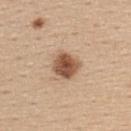Q: Was a biopsy performed?
A: no biopsy performed (imaged during a skin exam)
Q: What lighting was used for the tile?
A: white-light
Q: Patient demographics?
A: female, in their mid-40s
Q: What kind of image is this?
A: 15 mm crop, total-body photography
Q: What is the lesion's diameter?
A: ≈3.5 mm
Q: What did automated image analysis measure?
A: a mean CIELAB color near L≈54 a*≈20 b*≈32 and a lesion–skin lightness drop of about 16; a border-irregularity rating of about 1.5/10, a color-variation rating of about 4.5/10, and peripheral color asymmetry of about 1.5; an automated nevus-likeness rating near 100 out of 100 and a detector confidence of about 100 out of 100 that the crop contains a lesion
Q: What is the anatomic site?
A: the back